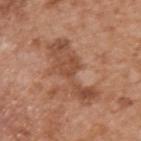Notes:
* notes · total-body-photography surveillance lesion; no biopsy